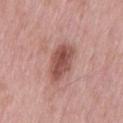Captured during whole-body skin photography for melanoma surveillance; the lesion was not biopsied.
Imaged with white-light lighting.
A male patient, aged approximately 55.
The lesion's longest dimension is about 4.5 mm.
A 15 mm close-up extracted from a 3D total-body photography capture.
Located on the leg.